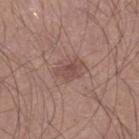Clinical impression:
The lesion was photographed on a routine skin check and not biopsied; there is no pathology result.
Clinical summary:
Cropped from a total-body skin-imaging series; the visible field is about 15 mm. The lesion-visualizer software estimated two-axis asymmetry of about 0.35. It also reported a border-irregularity index near 3.5/10, a color-variation rating of about 2.5/10, and radial color variation of about 1. The software also gave an automated nevus-likeness rating near 5 out of 100 and a detector confidence of about 100 out of 100 that the crop contains a lesion. The lesion is on the right lower leg. A male subject approximately 25 years of age. The recorded lesion diameter is about 3.5 mm. Imaged with white-light lighting.